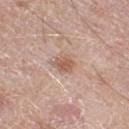Impression:
Recorded during total-body skin imaging; not selected for excision or biopsy.
Background:
A male subject aged approximately 80. On the right thigh. Cropped from a whole-body photographic skin survey; the tile spans about 15 mm.